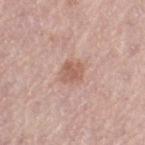The lesion was tiled from a total-body skin photograph and was not biopsied.
This is a white-light tile.
The subject is a female aged approximately 65.
An algorithmic analysis of the crop reported a lesion color around L≈58 a*≈21 b*≈27 in CIELAB and a lesion–skin lightness drop of about 9. It also reported an automated nevus-likeness rating near 50 out of 100.
Longest diameter approximately 2.5 mm.
A lesion tile, about 15 mm wide, cut from a 3D total-body photograph.
On the left thigh.